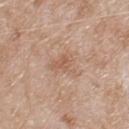biopsy_status: not biopsied; imaged during a skin examination
patient:
  sex: male
  age_approx: 80
image:
  source: total-body photography crop
  field_of_view_mm: 15
lesion_size:
  long_diameter_mm_approx: 4.5
site: back